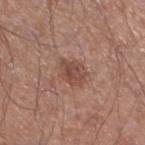This lesion was catalogued during total-body skin photography and was not selected for biopsy.
On the left lower leg.
The patient is a male aged 53 to 57.
A roughly 15 mm field-of-view crop from a total-body skin photograph.
Captured under white-light illumination.
An algorithmic analysis of the crop reported a lesion area of about 7.5 mm² and a symmetry-axis asymmetry near 0.2. The analysis additionally found a lesion color around L≈48 a*≈21 b*≈26 in CIELAB, roughly 9 lightness units darker than nearby skin, and a lesion-to-skin contrast of about 7 (normalized; higher = more distinct). The software also gave a peripheral color-asymmetry measure near 1.5.